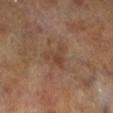A 15 mm crop from a total-body photograph taken for skin-cancer surveillance. The patient is a male about 65 years old. The total-body-photography lesion software estimated an average lesion color of about L≈41 a*≈17 b*≈29 (CIELAB) and roughly 6 lightness units darker than nearby skin. The analysis additionally found border irregularity of about 6.5 on a 0–10 scale, a within-lesion color-variation index near 2.5/10, and peripheral color asymmetry of about 1. The software also gave a classifier nevus-likeness of about 0/100 and lesion-presence confidence of about 100/100. Imaged with cross-polarized lighting. The recorded lesion diameter is about 3 mm.